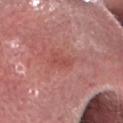follow-up: catalogued during a skin exam; not biopsied
lighting: white-light illumination
lesion size: about 2.5 mm
imaging modality: ~15 mm tile from a whole-body skin photo
site: the head or neck
patient: male, aged 63 to 67